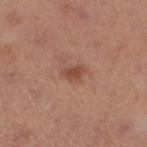This lesion was catalogued during total-body skin photography and was not selected for biopsy. On the left thigh. A female patient about 40 years old. A close-up tile cropped from a whole-body skin photograph, about 15 mm across.